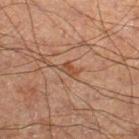Q: Was a biopsy performed?
A: imaged on a skin check; not biopsied
Q: Automated lesion metrics?
A: a border-irregularity index near 4.5/10, a color-variation rating of about 1.5/10, and radial color variation of about 0.5; a nevus-likeness score of about 0/100 and a lesion-detection confidence of about 100/100
Q: Lesion location?
A: the right lower leg
Q: How was the tile lit?
A: cross-polarized
Q: What are the patient's age and sex?
A: male, approximately 65 years of age
Q: How was this image acquired?
A: total-body-photography crop, ~15 mm field of view
Q: What is the lesion's diameter?
A: about 2.5 mm